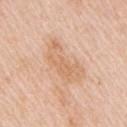The lesion was tiled from a total-body skin photograph and was not biopsied.
A female patient about 45 years old.
This image is a 15 mm lesion crop taken from a total-body photograph.
Imaged with white-light lighting.
Located on the right upper arm.